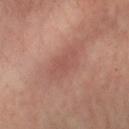Impression:
The lesion was tiled from a total-body skin photograph and was not biopsied.
Acquisition and patient details:
Automated tile analysis of the lesion measured a footprint of about 7.5 mm² and an eccentricity of roughly 0.9. And it measured a mean CIELAB color near L≈51 a*≈23 b*≈25 and a normalized lesion–skin contrast near 4.5. The software also gave an automated nevus-likeness rating near 0 out of 100 and a lesion-detection confidence of about 100/100. A male subject, in their 50s. A region of skin cropped from a whole-body photographic capture, roughly 15 mm wide. Located on the left forearm. About 4.5 mm across. The tile uses cross-polarized illumination.